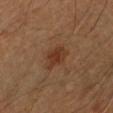notes: catalogued during a skin exam; not biopsied
acquisition: ~15 mm crop, total-body skin-cancer survey
illumination: cross-polarized illumination
image-analysis metrics: a lesion area of about 5 mm², a shape eccentricity near 0.7, and two-axis asymmetry of about 0.25
patient: male, in their 60s
location: the right upper arm
lesion size: about 3.5 mm